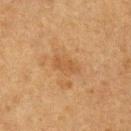Q: What lighting was used for the tile?
A: cross-polarized
Q: What is the imaging modality?
A: ~15 mm tile from a whole-body skin photo
Q: Who is the patient?
A: female, roughly 55 years of age
Q: What is the anatomic site?
A: the left upper arm